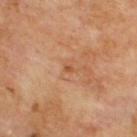follow-up: catalogued during a skin exam; not biopsied
lesion diameter: ~1 mm (longest diameter)
image: total-body-photography crop, ~15 mm field of view
lighting: cross-polarized illumination
image-analysis metrics: a lesion color around L≈50 a*≈24 b*≈36 in CIELAB, about 8 CIELAB-L* units darker than the surrounding skin, and a normalized border contrast of about 6.5; an automated nevus-likeness rating near 0 out of 100
anatomic site: the upper back
subject: male, in their 70s